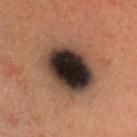Q: What is the imaging modality?
A: ~15 mm tile from a whole-body skin photo
Q: What lighting was used for the tile?
A: cross-polarized illumination
Q: What is the lesion's diameter?
A: ≈6.5 mm
Q: Who is the patient?
A: male, approximately 35 years of age
Q: Lesion location?
A: the head or neck